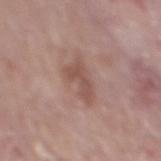Imaged during a routine full-body skin examination; the lesion was not biopsied and no histopathology is available.
A male subject, approximately 70 years of age.
Cropped from a total-body skin-imaging series; the visible field is about 15 mm.
On the mid back.
Automated image analysis of the tile measured border irregularity of about 5 on a 0–10 scale, internal color variation of about 2 on a 0–10 scale, and peripheral color asymmetry of about 0.5. It also reported an automated nevus-likeness rating near 0 out of 100 and a detector confidence of about 100 out of 100 that the crop contains a lesion.
Approximately 4.5 mm at its widest.
Imaged with white-light lighting.